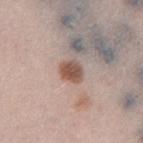The lesion was tiled from a total-body skin photograph and was not biopsied.
Cropped from a total-body skin-imaging series; the visible field is about 15 mm.
Imaged with white-light lighting.
The recorded lesion diameter is about 3 mm.
The total-body-photography lesion software estimated a lesion color around L≈53 a*≈18 b*≈25 in CIELAB, about 13 CIELAB-L* units darker than the surrounding skin, and a normalized border contrast of about 9. The software also gave a classifier nevus-likeness of about 100/100 and lesion-presence confidence of about 100/100.
A male patient, in their 30s.